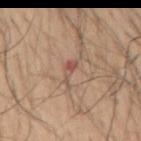This lesion was catalogued during total-body skin photography and was not selected for biopsy. A close-up tile cropped from a whole-body skin photograph, about 15 mm across. Imaged with white-light lighting. A male patient approximately 55 years of age. The recorded lesion diameter is about 3.5 mm. The lesion is located on the right upper arm.